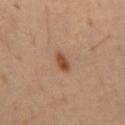Q: Is there a histopathology result?
A: imaged on a skin check; not biopsied
Q: Where on the body is the lesion?
A: the mid back
Q: How large is the lesion?
A: ≈3 mm
Q: Illumination type?
A: cross-polarized
Q: Who is the patient?
A: male, aged around 55
Q: What kind of image is this?
A: 15 mm crop, total-body photography